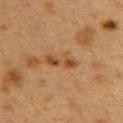A female subject, aged approximately 40. From the upper back. A 15 mm close-up tile from a total-body photography series done for melanoma screening.